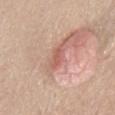Imaged during a routine full-body skin examination; the lesion was not biopsied and no histopathology is available.
The subject is a male roughly 80 years of age.
From the abdomen.
A 15 mm close-up tile from a total-body photography series done for melanoma screening.
The recorded lesion diameter is about 3.5 mm.